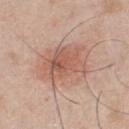  biopsy_status: not biopsied; imaged during a skin examination
  image:
    source: total-body photography crop
    field_of_view_mm: 15
  patient:
    sex: male
    age_approx: 45
  lighting: white-light
  site: chest